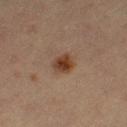biopsy status — no biopsy performed (imaged during a skin exam)
image-analysis metrics — a footprint of about 4.5 mm², an outline eccentricity of about 0.55 (0 = round, 1 = elongated), and a shape-asymmetry score of about 0.15 (0 = symmetric); an average lesion color of about L≈36 a*≈18 b*≈28 (CIELAB), roughly 10 lightness units darker than nearby skin, and a lesion-to-skin contrast of about 10 (normalized; higher = more distinct); a border-irregularity index near 1.5/10, a within-lesion color-variation index near 5/10, and peripheral color asymmetry of about 1.5; an automated nevus-likeness rating near 100 out of 100
subject — female, aged approximately 30
lesion diameter — ≈2.5 mm
imaging modality — total-body-photography crop, ~15 mm field of view
anatomic site — the left thigh